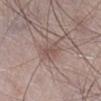<tbp_lesion>
<biopsy_status>not biopsied; imaged during a skin examination</biopsy_status>
<patient>
  <sex>male</sex>
  <age_approx>65</age_approx>
</patient>
<site>right lower leg</site>
<image>
  <source>total-body photography crop</source>
  <field_of_view_mm>15</field_of_view_mm>
</image>
<lesion_size>
  <long_diameter_mm_approx>3.0</long_diameter_mm_approx>
</lesion_size>
<lighting>white-light</lighting>
<automated_metrics>
  <area_mm2_approx>3.5</area_mm2_approx>
  <eccentricity>0.9</eccentricity>
  <shape_asymmetry>0.35</shape_asymmetry>
  <border_irregularity_0_10>4.0</border_irregularity_0_10>
  <color_variation_0_10>0.0</color_variation_0_10>
  <peripheral_color_asymmetry>0.0</peripheral_color_asymmetry>
</automated_metrics>
</tbp_lesion>